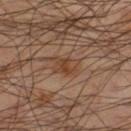• biopsy status: total-body-photography surveillance lesion; no biopsy
• site: the leg
• imaging modality: ~15 mm tile from a whole-body skin photo
• image-analysis metrics: a lesion color around L≈42 a*≈20 b*≈31 in CIELAB, a lesion–skin lightness drop of about 7, and a lesion-to-skin contrast of about 7 (normalized; higher = more distinct); border irregularity of about 2.5 on a 0–10 scale and a peripheral color-asymmetry measure near 1
• subject: male, aged approximately 50
• lesion diameter: about 2.5 mm